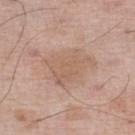notes — no biopsy performed (imaged during a skin exam)
lighting — white-light
imaging modality — 15 mm crop, total-body photography
anatomic site — the leg
automated metrics — internal color variation of about 2.5 on a 0–10 scale
diameter — ≈5.5 mm
subject — male, aged around 70